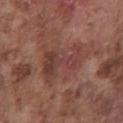The lesion was tiled from a total-body skin photograph and was not biopsied. A close-up tile cropped from a whole-body skin photograph, about 15 mm across. A male subject approximately 75 years of age. Located on the chest.